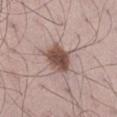Q: Was a biopsy performed?
A: no biopsy performed (imaged during a skin exam)
Q: Lesion location?
A: the left thigh
Q: How large is the lesion?
A: about 3.5 mm
Q: How was the tile lit?
A: white-light illumination
Q: What are the patient's age and sex?
A: male, roughly 70 years of age
Q: What did automated image analysis measure?
A: a mean CIELAB color near L≈50 a*≈17 b*≈23, a lesion–skin lightness drop of about 15, and a normalized border contrast of about 10.5
Q: How was this image acquired?
A: 15 mm crop, total-body photography